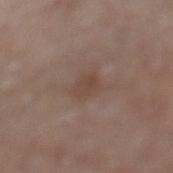notes = total-body-photography surveillance lesion; no biopsy | location = the leg | imaging modality = ~15 mm tile from a whole-body skin photo | lesion size = about 3 mm | TBP lesion metrics = an area of roughly 4.5 mm², an eccentricity of roughly 0.65, and a symmetry-axis asymmetry near 0.35; border irregularity of about 3 on a 0–10 scale and internal color variation of about 2 on a 0–10 scale | patient = male, roughly 65 years of age.